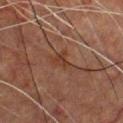Impression:
The lesion was tiled from a total-body skin photograph and was not biopsied.
Background:
Longest diameter approximately 3.5 mm. A male patient in their 50s. The lesion is on the front of the torso. A region of skin cropped from a whole-body photographic capture, roughly 15 mm wide. The total-body-photography lesion software estimated a lesion area of about 5.5 mm², an outline eccentricity of about 0.75 (0 = round, 1 = elongated), and a symmetry-axis asymmetry near 0.45. It also reported a mean CIELAB color near L≈30 a*≈17 b*≈23, about 5 CIELAB-L* units darker than the surrounding skin, and a normalized lesion–skin contrast near 6. And it measured border irregularity of about 5 on a 0–10 scale, internal color variation of about 2.5 on a 0–10 scale, and peripheral color asymmetry of about 0.5. It also reported lesion-presence confidence of about 90/100. The tile uses cross-polarized illumination.